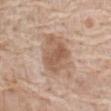Part of a total-body skin-imaging series; this lesion was reviewed on a skin check and was not flagged for biopsy.
A lesion tile, about 15 mm wide, cut from a 3D total-body photograph.
A female patient in their mid- to late 70s.
The lesion is located on the abdomen.